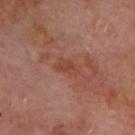Part of a total-body skin-imaging series; this lesion was reviewed on a skin check and was not flagged for biopsy. A close-up tile cropped from a whole-body skin photograph, about 15 mm across. On the upper back. This is a cross-polarized tile. A male subject, aged around 70.